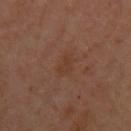Impression: No biopsy was performed on this lesion — it was imaged during a full skin examination and was not determined to be concerning. Background: A female patient roughly 60 years of age. The tile uses cross-polarized illumination. The lesion is located on the arm. The lesion's longest dimension is about 2.5 mm. A roughly 15 mm field-of-view crop from a total-body skin photograph.